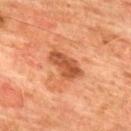The lesion was photographed on a routine skin check and not biopsied; there is no pathology result.
The recorded lesion diameter is about 4.5 mm.
The tile uses cross-polarized illumination.
From the upper back.
The lesion-visualizer software estimated a lesion area of about 8.5 mm², an outline eccentricity of about 0.85 (0 = round, 1 = elongated), and a symmetry-axis asymmetry near 0.25. It also reported a color-variation rating of about 3.5/10 and radial color variation of about 1.5.
The patient is a male aged 73–77.
A lesion tile, about 15 mm wide, cut from a 3D total-body photograph.